A 15 mm crop from a total-body photograph taken for skin-cancer surveillance; on the front of the torso; a male patient, aged 63–67 — 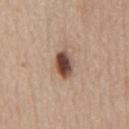On biopsy, histopathology showed a benign skin lesion: benign follicular adnexal tumor.